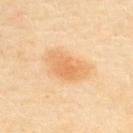notes: total-body-photography surveillance lesion; no biopsy | size: ≈6 mm | patient: female, aged approximately 40 | TBP lesion metrics: an eccentricity of roughly 0.8 and a shape-asymmetry score of about 0.25 (0 = symmetric); border irregularity of about 2.5 on a 0–10 scale, a within-lesion color-variation index near 4/10, and peripheral color asymmetry of about 1; an automated nevus-likeness rating near 90 out of 100 and lesion-presence confidence of about 100/100 | site: the upper back | imaging modality: total-body-photography crop, ~15 mm field of view | lighting: cross-polarized illumination.